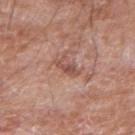A region of skin cropped from a whole-body photographic capture, roughly 15 mm wide. On the right upper arm. About 3 mm across. Captured under white-light illumination. A male subject, approximately 60 years of age. An algorithmic analysis of the crop reported about 9 CIELAB-L* units darker than the surrounding skin and a lesion-to-skin contrast of about 6.5 (normalized; higher = more distinct). The software also gave a classifier nevus-likeness of about 0/100.